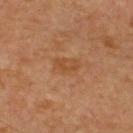biopsy status = catalogued during a skin exam; not biopsied
acquisition = ~15 mm crop, total-body skin-cancer survey
automated lesion analysis = border irregularity of about 2.5 on a 0–10 scale, a color-variation rating of about 3/10, and radial color variation of about 1
subject = male, aged around 60
illumination = cross-polarized
site = the upper back